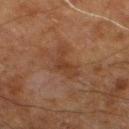<case>
<biopsy_status>not biopsied; imaged during a skin examination</biopsy_status>
</case>